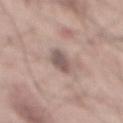TBP lesion metrics — an area of roughly 6 mm², a shape eccentricity near 0.85, and a shape-asymmetry score of about 0.3 (0 = symmetric); a lesion–skin lightness drop of about 12 and a normalized border contrast of about 8 | imaging modality — ~15 mm tile from a whole-body skin photo | lesion size — about 3.5 mm | patient — male, aged approximately 55 | location — the mid back.